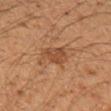Q: Is there a histopathology result?
A: imaged on a skin check; not biopsied
Q: What did automated image analysis measure?
A: a detector confidence of about 100 out of 100 that the crop contains a lesion
Q: What is the anatomic site?
A: the left upper arm
Q: Patient demographics?
A: male, in their 50s
Q: What kind of image is this?
A: ~15 mm tile from a whole-body skin photo
Q: What lighting was used for the tile?
A: cross-polarized illumination
Q: What is the lesion's diameter?
A: about 3 mm